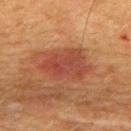Cropped from a total-body skin-imaging series; the visible field is about 15 mm. On the upper back. A male patient, in their 50s. This is a cross-polarized tile. Automated image analysis of the tile measured an area of roughly 17 mm², an eccentricity of roughly 0.7, and two-axis asymmetry of about 0.25. It also reported lesion-presence confidence of about 100/100.